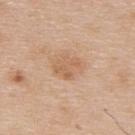No biopsy was performed on this lesion — it was imaged during a full skin examination and was not determined to be concerning. Located on the upper back. A male patient about 65 years old. A region of skin cropped from a whole-body photographic capture, roughly 15 mm wide. This is a white-light tile. Approximately 3.5 mm at its widest.